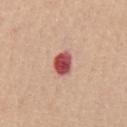Case summary:
• TBP lesion metrics · an automated nevus-likeness rating near 0 out of 100 and lesion-presence confidence of about 100/100
• lighting · white-light
• diameter · ≈3 mm
• acquisition · ~15 mm tile from a whole-body skin photo
• body site · the front of the torso
• patient · male, aged 63 to 67